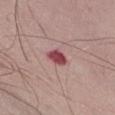Impression: This lesion was catalogued during total-body skin photography and was not selected for biopsy. Context: A male patient, in their mid- to late 60s. About 2.5 mm across. A roughly 15 mm field-of-view crop from a total-body skin photograph. On the front of the torso.